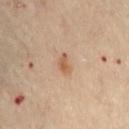Q: Is there a histopathology result?
A: total-body-photography surveillance lesion; no biopsy
Q: What are the patient's age and sex?
A: female, about 60 years old
Q: Illumination type?
A: cross-polarized
Q: Lesion location?
A: the abdomen
Q: What is the lesion's diameter?
A: about 2.5 mm
Q: How was this image acquired?
A: ~15 mm tile from a whole-body skin photo